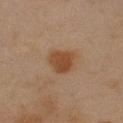* notes · no biopsy performed (imaged during a skin exam)
* anatomic site · the right upper arm
* patient · male, in their mid-40s
* lesion size · ~3 mm (longest diameter)
* lighting · cross-polarized
* imaging modality · total-body-photography crop, ~15 mm field of view